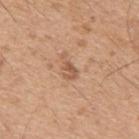Imaged during a routine full-body skin examination; the lesion was not biopsied and no histopathology is available.